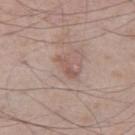workup: no biopsy performed (imaged during a skin exam)
site: the right thigh
image: ~15 mm tile from a whole-body skin photo
subject: male, about 55 years old
tile lighting: white-light